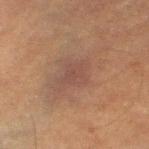Q: Is there a histopathology result?
A: total-body-photography surveillance lesion; no biopsy